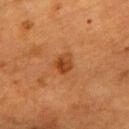| feature | finding |
|---|---|
| follow-up | imaged on a skin check; not biopsied |
| image source | 15 mm crop, total-body photography |
| subject | female, approximately 55 years of age |
| tile lighting | cross-polarized illumination |
| body site | the upper back |